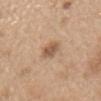image source: total-body-photography crop, ~15 mm field of view
tile lighting: white-light illumination
automated metrics: a footprint of about 4.5 mm², an outline eccentricity of about 0.75 (0 = round, 1 = elongated), and a symmetry-axis asymmetry near 0.25; a border-irregularity index near 2.5/10, internal color variation of about 2 on a 0–10 scale, and a peripheral color-asymmetry measure near 0.5
location: the abdomen
subject: male, roughly 70 years of age